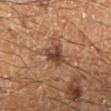biopsy_status: not biopsied; imaged during a skin examination
image:
  source: total-body photography crop
  field_of_view_mm: 15
patient:
  sex: male
  age_approx: 60
site: leg